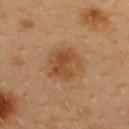The lesion was photographed on a routine skin check and not biopsied; there is no pathology result. A male patient aged approximately 40. The lesion is located on the upper back. The lesion's longest dimension is about 4 mm. Cropped from a total-body skin-imaging series; the visible field is about 15 mm.